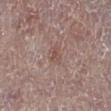Findings:
• site: the leg
• patient: female, about 65 years old
• size: ~2.5 mm (longest diameter)
• image: ~15 mm tile from a whole-body skin photo